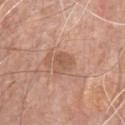Acquisition and patient details:
The patient is a male roughly 80 years of age. From the chest. Cropped from a whole-body photographic skin survey; the tile spans about 15 mm.